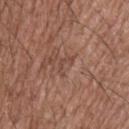acquisition — ~15 mm tile from a whole-body skin photo | TBP lesion metrics — a footprint of about 2.5 mm² and a shape eccentricity near 0.8; a mean CIELAB color near L≈46 a*≈20 b*≈26, about 6 CIELAB-L* units darker than the surrounding skin, and a lesion-to-skin contrast of about 5 (normalized; higher = more distinct); a border-irregularity index near 7.5/10 and a peripheral color-asymmetry measure near 0 | location — the back | subject — male, aged 63 to 67.